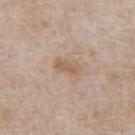Q: Is there a histopathology result?
A: imaged on a skin check; not biopsied
Q: Automated lesion metrics?
A: a shape eccentricity near 0.9 and a symmetry-axis asymmetry near 0.25; a border-irregularity index near 3/10 and a within-lesion color-variation index near 0.5/10
Q: How was the tile lit?
A: white-light illumination
Q: Who is the patient?
A: male, in their mid-60s
Q: How was this image acquired?
A: total-body-photography crop, ~15 mm field of view
Q: How large is the lesion?
A: about 3 mm
Q: What is the anatomic site?
A: the abdomen